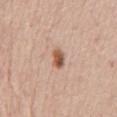biopsy_status: not biopsied; imaged during a skin examination
site: abdomen
patient:
  sex: female
  age_approx: 70
automated_metrics:
  area_mm2_approx: 4.0
  shape_asymmetry: 0.2
  cielab_L: 56
  cielab_a: 21
  cielab_b: 31
  vs_skin_darker_L: 14.0
image:
  source: total-body photography crop
  field_of_view_mm: 15
lesion_size:
  long_diameter_mm_approx: 2.5